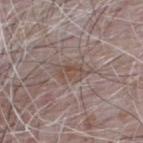biopsy status: no biopsy performed (imaged during a skin exam); diameter: ~3.5 mm (longest diameter); acquisition: ~15 mm tile from a whole-body skin photo; location: the upper back; subject: male, aged around 65; TBP lesion metrics: a shape eccentricity near 0.85 and a symmetry-axis asymmetry near 0.3; lighting: white-light illumination.